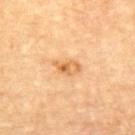<case>
  <biopsy_status>not biopsied; imaged during a skin examination</biopsy_status>
  <patient>
    <sex>male</sex>
    <age_approx>85+</age_approx>
  </patient>
  <site>upper back</site>
  <image>
    <source>total-body photography crop</source>
    <field_of_view_mm>15</field_of_view_mm>
  </image>
</case>